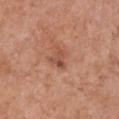Recorded during total-body skin imaging; not selected for excision or biopsy. Measured at roughly 2.5 mm in maximum diameter. The lesion-visualizer software estimated a lesion area of about 3.5 mm², a shape eccentricity near 0.65, and a shape-asymmetry score of about 0.6 (0 = symmetric). The analysis additionally found a lesion color around L≈50 a*≈24 b*≈30 in CIELAB and roughly 9 lightness units darker than nearby skin. The analysis additionally found a border-irregularity index near 5.5/10, internal color variation of about 4 on a 0–10 scale, and peripheral color asymmetry of about 1.5. The analysis additionally found a classifier nevus-likeness of about 5/100 and a detector confidence of about 100 out of 100 that the crop contains a lesion. The patient is a female about 65 years old. The tile uses white-light illumination. From the front of the torso. Cropped from a total-body skin-imaging series; the visible field is about 15 mm.